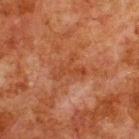The lesion was photographed on a routine skin check and not biopsied; there is no pathology result.
Located on the back.
The subject is a male in their 80s.
A lesion tile, about 15 mm wide, cut from a 3D total-body photograph.